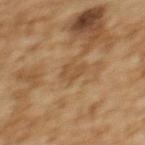follow-up: catalogued during a skin exam; not biopsied | tile lighting: cross-polarized | subject: female, aged 58–62 | automated metrics: a footprint of about 4 mm², a shape eccentricity near 0.8, and a shape-asymmetry score of about 0.4 (0 = symmetric); a lesion–skin lightness drop of about 6 and a normalized lesion–skin contrast near 5.5 | image: total-body-photography crop, ~15 mm field of view | location: the upper back.